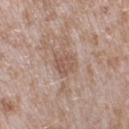Part of a total-body skin-imaging series; this lesion was reviewed on a skin check and was not flagged for biopsy. Located on the right upper arm. Cropped from a total-body skin-imaging series; the visible field is about 15 mm. A male patient aged around 45.